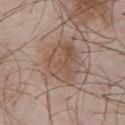Clinical impression:
The lesion was photographed on a routine skin check and not biopsied; there is no pathology result.
Image and clinical context:
A roughly 15 mm field-of-view crop from a total-body skin photograph. The tile uses white-light illumination. The lesion is located on the chest. The recorded lesion diameter is about 5 mm. The patient is a male aged around 55.